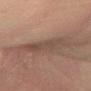patient: male, in their 70s | site: the left forearm | image: ~15 mm tile from a whole-body skin photo.